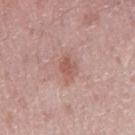follow-up=imaged on a skin check; not biopsied | acquisition=~15 mm crop, total-body skin-cancer survey | location=the arm | tile lighting=white-light illumination | patient=male, aged approximately 50 | diameter=~3 mm (longest diameter) | automated metrics=a footprint of about 4 mm² and an eccentricity of roughly 0.8; a lesion-detection confidence of about 100/100.